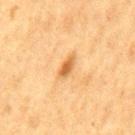The lesion was tiled from a total-body skin photograph and was not biopsied. A male subject, about 75 years old. From the mid back. A roughly 15 mm field-of-view crop from a total-body skin photograph.